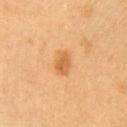No biopsy was performed on this lesion — it was imaged during a full skin examination and was not determined to be concerning. The lesion is located on the left upper arm. Measured at roughly 3 mm in maximum diameter. An algorithmic analysis of the crop reported a mean CIELAB color near L≈56 a*≈23 b*≈41, a lesion–skin lightness drop of about 10, and a lesion-to-skin contrast of about 7 (normalized; higher = more distinct). It also reported a nevus-likeness score of about 80/100 and a detector confidence of about 100 out of 100 that the crop contains a lesion. The tile uses cross-polarized illumination. The patient is a female in their 30s. A 15 mm crop from a total-body photograph taken for skin-cancer surveillance.